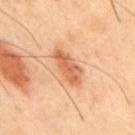The lesion was photographed on a routine skin check and not biopsied; there is no pathology result. A roughly 15 mm field-of-view crop from a total-body skin photograph. Longest diameter approximately 5.5 mm. Imaged with cross-polarized lighting. The lesion-visualizer software estimated an eccentricity of roughly 0.9. It also reported a lesion–skin lightness drop of about 11 and a normalized border contrast of about 7. It also reported a border-irregularity rating of about 3/10, a color-variation rating of about 3.5/10, and peripheral color asymmetry of about 1. A male subject, aged around 45. The lesion is on the mid back.